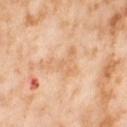Assessment: The lesion was tiled from a total-body skin photograph and was not biopsied. Context: The lesion is on the right thigh. The subject is a female aged approximately 55. A roughly 15 mm field-of-view crop from a total-body skin photograph.